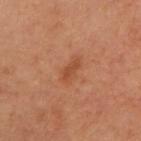<lesion>
<biopsy_status>not biopsied; imaged during a skin examination</biopsy_status>
<lighting>cross-polarized</lighting>
<patient>
  <sex>female</sex>
  <age_approx>55</age_approx>
</patient>
<image>
  <source>total-body photography crop</source>
  <field_of_view_mm>15</field_of_view_mm>
</image>
<site>front of the torso</site>
</lesion>